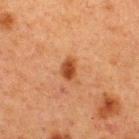This lesion was catalogued during total-body skin photography and was not selected for biopsy.
Imaged with cross-polarized lighting.
Measured at roughly 2.5 mm in maximum diameter.
A region of skin cropped from a whole-body photographic capture, roughly 15 mm wide.
The lesion is located on the upper back.
A male subject roughly 60 years of age.
The total-body-photography lesion software estimated an area of roughly 4 mm², a shape eccentricity near 0.7, and a shape-asymmetry score of about 0.25 (0 = symmetric). And it measured a nevus-likeness score of about 95/100 and a lesion-detection confidence of about 100/100.